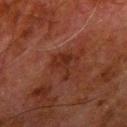Case summary:
* notes — no biopsy performed (imaged during a skin exam)
* automated metrics — about 5 CIELAB-L* units darker than the surrounding skin and a normalized lesion–skin contrast near 6.5; a border-irregularity index near 7.5/10, internal color variation of about 2 on a 0–10 scale, and peripheral color asymmetry of about 1
* image — 15 mm crop, total-body photography
* site — the right upper arm
* lighting — cross-polarized illumination
* patient — male, aged 78 to 82